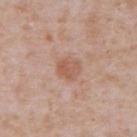The lesion was photographed on a routine skin check and not biopsied; there is no pathology result.
Approximately 3 mm at its widest.
A male patient, aged 63 to 67.
A 15 mm close-up tile from a total-body photography series done for melanoma screening.
Located on the abdomen.
The tile uses white-light illumination.
The lesion-visualizer software estimated a nevus-likeness score of about 5/100 and a detector confidence of about 100 out of 100 that the crop contains a lesion.